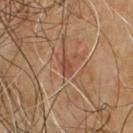The lesion was tiled from a total-body skin photograph and was not biopsied.
A male subject, aged approximately 65.
A 15 mm crop from a total-body photograph taken for skin-cancer surveillance.
The tile uses cross-polarized illumination.
Approximately 2.5 mm at its widest.